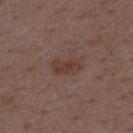The lesion was tiled from a total-body skin photograph and was not biopsied.
Located on the mid back.
This image is a 15 mm lesion crop taken from a total-body photograph.
Captured under white-light illumination.
A male patient, aged 48–52.